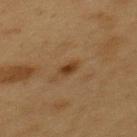workup=catalogued during a skin exam; not biopsied
site=the mid back
diameter=about 2.5 mm
imaging modality=total-body-photography crop, ~15 mm field of view
subject=male, about 55 years old
tile lighting=cross-polarized